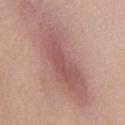Q: Was this lesion biopsied?
A: catalogued during a skin exam; not biopsied
Q: What lighting was used for the tile?
A: white-light illumination
Q: What did automated image analysis measure?
A: an automated nevus-likeness rating near 40 out of 100 and a lesion-detection confidence of about 55/100
Q: What is the lesion's diameter?
A: about 10.5 mm
Q: How was this image acquired?
A: total-body-photography crop, ~15 mm field of view
Q: Who is the patient?
A: female, aged 38 to 42
Q: What is the anatomic site?
A: the chest